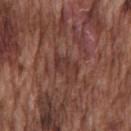Findings:
• follow-up · total-body-photography surveillance lesion; no biopsy
• anatomic site · the chest
• imaging modality · total-body-photography crop, ~15 mm field of view
• patient · male, about 75 years old
• lighting · white-light
• size · ~3 mm (longest diameter)
• TBP lesion metrics · a classifier nevus-likeness of about 0/100 and lesion-presence confidence of about 65/100